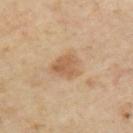  biopsy_status: not biopsied; imaged during a skin examination
  image:
    source: total-body photography crop
    field_of_view_mm: 15
  site: upper back
  patient:
    age_approx: 55